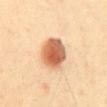The lesion was photographed on a routine skin check and not biopsied; there is no pathology result.
Imaged with cross-polarized lighting.
A male patient about 40 years old.
The recorded lesion diameter is about 4.5 mm.
A 15 mm close-up tile from a total-body photography series done for melanoma screening.
The lesion is located on the front of the torso.